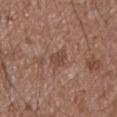Findings:
• biopsy status — total-body-photography surveillance lesion; no biopsy
• subject — male, roughly 75 years of age
• image-analysis metrics — a color-variation rating of about 1.5/10; a nevus-likeness score of about 0/100 and a detector confidence of about 100 out of 100 that the crop contains a lesion
• lesion diameter — about 2.5 mm
• acquisition — ~15 mm tile from a whole-body skin photo
• illumination — white-light illumination
• location — the front of the torso